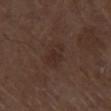No biopsy was performed on this lesion — it was imaged during a full skin examination and was not determined to be concerning. A roughly 15 mm field-of-view crop from a total-body skin photograph. The patient is a male approximately 75 years of age. Automated image analysis of the tile measured an area of roughly 5.5 mm² and a shape eccentricity near 0.8. And it measured a lesion color around L≈27 a*≈16 b*≈21 in CIELAB and about 5 CIELAB-L* units darker than the surrounding skin. It also reported a nevus-likeness score of about 0/100 and lesion-presence confidence of about 100/100. The recorded lesion diameter is about 3.5 mm. On the right thigh.